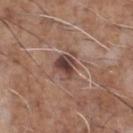{
  "biopsy_status": "not biopsied; imaged during a skin examination",
  "image": {
    "source": "total-body photography crop",
    "field_of_view_mm": 15
  },
  "patient": {
    "sex": "male",
    "age_approx": 70
  },
  "site": "chest",
  "automated_metrics": {
    "area_mm2_approx": 7.5,
    "shape_asymmetry": 0.3,
    "cielab_L": 44,
    "cielab_a": 18,
    "cielab_b": 23,
    "vs_skin_darker_L": 12.0,
    "nevus_likeness_0_100": 5,
    "lesion_detection_confidence_0_100": 100
  },
  "lighting": "white-light"
}